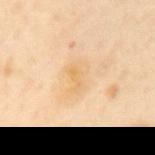notes = catalogued during a skin exam; not biopsied
site = the mid back
subject = male, approximately 65 years of age
image = 15 mm crop, total-body photography
lighting = cross-polarized